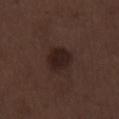workup=catalogued during a skin exam; not biopsied
location=the leg
lighting=white-light illumination
acquisition=~15 mm tile from a whole-body skin photo
lesion diameter=about 3.5 mm
subject=male, aged 68 to 72